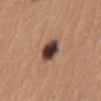Impression:
This lesion was catalogued during total-body skin photography and was not selected for biopsy.
Background:
Cropped from a total-body skin-imaging series; the visible field is about 15 mm. The subject is a female approximately 40 years of age. Located on the abdomen.